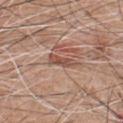workup = imaged on a skin check; not biopsied
subject = male, aged around 60
automated metrics = an area of roughly 4.5 mm², an eccentricity of roughly 0.95, and a symmetry-axis asymmetry near 0.35
anatomic site = the chest
imaging modality = total-body-photography crop, ~15 mm field of view
lesion diameter = about 3.5 mm